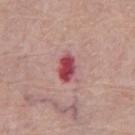Impression: Part of a total-body skin-imaging series; this lesion was reviewed on a skin check and was not flagged for biopsy. Background: A male patient, approximately 80 years of age. Located on the abdomen. The recorded lesion diameter is about 4 mm. Imaged with white-light lighting. Cropped from a whole-body photographic skin survey; the tile spans about 15 mm.